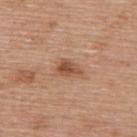Imaged during a routine full-body skin examination; the lesion was not biopsied and no histopathology is available.
The patient is a female aged approximately 60.
Measured at roughly 3 mm in maximum diameter.
Captured under white-light illumination.
From the upper back.
Automated image analysis of the tile measured an average lesion color of about L≈51 a*≈22 b*≈32 (CIELAB), about 12 CIELAB-L* units darker than the surrounding skin, and a lesion-to-skin contrast of about 8 (normalized; higher = more distinct).
A 15 mm close-up extracted from a 3D total-body photography capture.